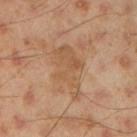No biopsy was performed on this lesion — it was imaged during a full skin examination and was not determined to be concerning. A male subject, in their mid- to late 40s. Captured under cross-polarized illumination. A region of skin cropped from a whole-body photographic capture, roughly 15 mm wide. On the right upper arm. The total-body-photography lesion software estimated roughly 7 lightness units darker than nearby skin and a normalized border contrast of about 5.5.